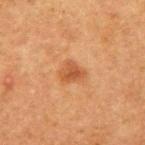The lesion was photographed on a routine skin check and not biopsied; there is no pathology result. A region of skin cropped from a whole-body photographic capture, roughly 15 mm wide. The tile uses cross-polarized illumination. The total-body-photography lesion software estimated a symmetry-axis asymmetry near 0.3. It also reported an average lesion color of about L≈43 a*≈23 b*≈34 (CIELAB) and a normalized lesion–skin contrast near 6.5. And it measured border irregularity of about 2.5 on a 0–10 scale, a within-lesion color-variation index near 2.5/10, and radial color variation of about 1. The analysis additionally found lesion-presence confidence of about 100/100. The patient is a male in their mid- to late 70s. The lesion's longest dimension is about 2.5 mm. The lesion is on the right upper arm.